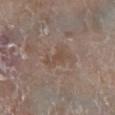{
  "biopsy_status": "not biopsied; imaged during a skin examination",
  "lighting": "white-light",
  "image": {
    "source": "total-body photography crop",
    "field_of_view_mm": 15
  },
  "lesion_size": {
    "long_diameter_mm_approx": 3.5
  },
  "patient": {
    "sex": "female",
    "age_approx": "85+"
  },
  "site": "leg"
}